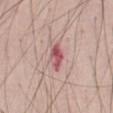Case summary:
– workup — imaged on a skin check; not biopsied
– automated lesion analysis — an average lesion color of about L≈54 a*≈30 b*≈20 (CIELAB), roughly 14 lightness units darker than nearby skin, and a normalized border contrast of about 9
– lighting — white-light
– lesion size — ≈3.5 mm
– patient — male, approximately 55 years of age
– anatomic site — the abdomen
– image source — ~15 mm tile from a whole-body skin photo